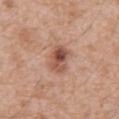anatomic site: the mid back | imaging modality: ~15 mm crop, total-body skin-cancer survey | patient: male, aged around 60.